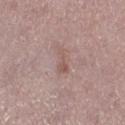Impression:
Imaged during a routine full-body skin examination; the lesion was not biopsied and no histopathology is available.
Clinical summary:
The patient is a female aged 48–52. The lesion-visualizer software estimated a footprint of about 3.5 mm², an outline eccentricity of about 0.9 (0 = round, 1 = elongated), and a shape-asymmetry score of about 0.5 (0 = symmetric). And it measured about 7 CIELAB-L* units darker than the surrounding skin and a normalized border contrast of about 5.5. The recorded lesion diameter is about 3 mm. This is a white-light tile. A close-up tile cropped from a whole-body skin photograph, about 15 mm across. From the leg.